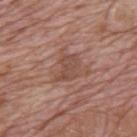| key | value |
|---|---|
| workup | no biopsy performed (imaged during a skin exam) |
| body site | the mid back |
| image | total-body-photography crop, ~15 mm field of view |
| patient | male, aged 68 to 72 |
| diameter | ~3.5 mm (longest diameter) |
| image-analysis metrics | about 7 CIELAB-L* units darker than the surrounding skin and a lesion-to-skin contrast of about 6 (normalized; higher = more distinct); a border-irregularity rating of about 3.5/10, internal color variation of about 1.5 on a 0–10 scale, and a peripheral color-asymmetry measure near 0.5 |